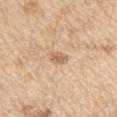follow-up: imaged on a skin check; not biopsied | lighting: white-light | acquisition: ~15 mm crop, total-body skin-cancer survey | anatomic site: the left upper arm | lesion diameter: ≈3 mm | image-analysis metrics: an eccentricity of roughly 0.85 and a symmetry-axis asymmetry near 0.25; a lesion-to-skin contrast of about 6 (normalized; higher = more distinct); a border-irregularity index near 2.5/10, internal color variation of about 2 on a 0–10 scale, and radial color variation of about 0.5; a nevus-likeness score of about 0/100 and a detector confidence of about 100 out of 100 that the crop contains a lesion | subject: male, aged around 70.